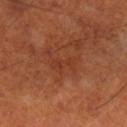Part of a total-body skin-imaging series; this lesion was reviewed on a skin check and was not flagged for biopsy.
A close-up tile cropped from a whole-body skin photograph, about 15 mm across.
A male subject roughly 70 years of age.
The lesion is on the right lower leg.